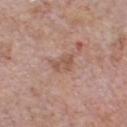Q: Was this lesion biopsied?
A: total-body-photography surveillance lesion; no biopsy
Q: Where on the body is the lesion?
A: the front of the torso
Q: How was the tile lit?
A: white-light illumination
Q: What kind of image is this?
A: ~15 mm crop, total-body skin-cancer survey
Q: What is the lesion's diameter?
A: ~2.5 mm (longest diameter)
Q: What are the patient's age and sex?
A: male, aged 58–62
Q: Automated lesion metrics?
A: an area of roughly 4 mm², an outline eccentricity of about 0.75 (0 = round, 1 = elongated), and a shape-asymmetry score of about 0.35 (0 = symmetric); a lesion color around L≈54 a*≈20 b*≈28 in CIELAB and a normalized border contrast of about 6; a classifier nevus-likeness of about 0/100 and a lesion-detection confidence of about 100/100